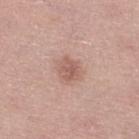workup: total-body-photography surveillance lesion; no biopsy
site: the left lower leg
patient: female, approximately 65 years of age
image: ~15 mm tile from a whole-body skin photo
lesion size: ≈2.5 mm
tile lighting: white-light
TBP lesion metrics: a footprint of about 5 mm² and a shape eccentricity near 0.5